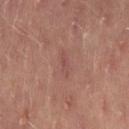Q: Who is the patient?
A: female, about 50 years old
Q: What is the anatomic site?
A: the right thigh
Q: Automated lesion metrics?
A: a lesion area of about 2.5 mm² and an eccentricity of roughly 0.95; an average lesion color of about L≈46 a*≈22 b*≈22 (CIELAB), about 6 CIELAB-L* units darker than the surrounding skin, and a normalized border contrast of about 4.5; a nevus-likeness score of about 0/100 and a detector confidence of about 100 out of 100 that the crop contains a lesion
Q: How large is the lesion?
A: ≈3 mm
Q: How was this image acquired?
A: total-body-photography crop, ~15 mm field of view
Q: Illumination type?
A: cross-polarized